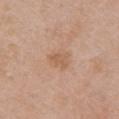Imaged during a routine full-body skin examination; the lesion was not biopsied and no histopathology is available. Captured under white-light illumination. The recorded lesion diameter is about 2.5 mm. The patient is a female in their 40s. Automated tile analysis of the lesion measured a lesion area of about 3 mm². And it measured an average lesion color of about L≈58 a*≈20 b*≈33 (CIELAB) and a lesion–skin lightness drop of about 7. The analysis additionally found lesion-presence confidence of about 100/100. From the chest. A region of skin cropped from a whole-body photographic capture, roughly 15 mm wide.